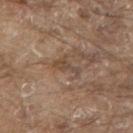The lesion was tiled from a total-body skin photograph and was not biopsied. Cropped from a total-body skin-imaging series; the visible field is about 15 mm. The tile uses white-light illumination. The recorded lesion diameter is about 3.5 mm. The lesion is located on the upper back. A male subject aged 78–82.